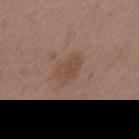Q: Was this lesion biopsied?
A: catalogued during a skin exam; not biopsied
Q: Patient demographics?
A: male, aged 48 to 52
Q: Lesion size?
A: ≈3.5 mm
Q: What kind of image is this?
A: 15 mm crop, total-body photography
Q: Lesion location?
A: the upper back